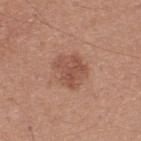Assessment: The lesion was photographed on a routine skin check and not biopsied; there is no pathology result. Image and clinical context: A male subject roughly 30 years of age. The lesion is on the upper back. A 15 mm close-up tile from a total-body photography series done for melanoma screening. Longest diameter approximately 4 mm. This is a white-light tile.